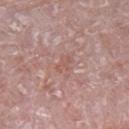The lesion was photographed on a routine skin check and not biopsied; there is no pathology result.
Captured under white-light illumination.
A male subject roughly 75 years of age.
This image is a 15 mm lesion crop taken from a total-body photograph.
From the left lower leg.
Automated image analysis of the tile measured an average lesion color of about L≈55 a*≈22 b*≈25 (CIELAB), a lesion–skin lightness drop of about 6, and a normalized border contrast of about 4.5. The analysis additionally found a nevus-likeness score of about 0/100.